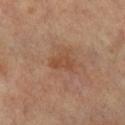• biopsy status — total-body-photography surveillance lesion; no biopsy
• site — the left leg
• image source — ~15 mm crop, total-body skin-cancer survey
• diameter — about 3 mm
• illumination — cross-polarized illumination
• patient — female, in their mid-60s
• automated lesion analysis — an area of roughly 4.5 mm², a shape eccentricity near 0.8, and a shape-asymmetry score of about 0.4 (0 = symmetric); a border-irregularity rating of about 3.5/10, a color-variation rating of about 2.5/10, and a peripheral color-asymmetry measure near 1; a classifier nevus-likeness of about 0/100